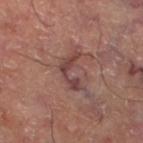workup: imaged on a skin check; not biopsied | anatomic site: the left thigh | image: ~15 mm tile from a whole-body skin photo | image-analysis metrics: an outline eccentricity of about 0.9 (0 = round, 1 = elongated) and two-axis asymmetry of about 0.65 | illumination: cross-polarized | size: about 4.5 mm.